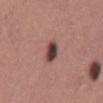Notes:
- follow-up · imaged on a skin check; not biopsied
- anatomic site · the mid back
- lesion size · ≈3 mm
- tile lighting · white-light
- subject · male, aged 43 to 47
- image-analysis metrics · a lesion color around L≈41 a*≈21 b*≈20 in CIELAB and a lesion-to-skin contrast of about 13 (normalized; higher = more distinct)
- image source · total-body-photography crop, ~15 mm field of view